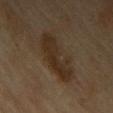Captured during whole-body skin photography for melanoma surveillance; the lesion was not biopsied. A roughly 15 mm field-of-view crop from a total-body skin photograph. The total-body-photography lesion software estimated an outline eccentricity of about 0.9 (0 = round, 1 = elongated) and a symmetry-axis asymmetry near 0.3. And it measured an average lesion color of about L≈26 a*≈11 b*≈23 (CIELAB) and a lesion–skin lightness drop of about 7. And it measured a color-variation rating of about 3/10 and a peripheral color-asymmetry measure near 1. The analysis additionally found lesion-presence confidence of about 95/100. A female patient, aged 58–62. Measured at roughly 7 mm in maximum diameter. From the chest.